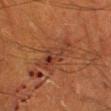<case>
<biopsy_status>not biopsied; imaged during a skin examination</biopsy_status>
<lighting>cross-polarized</lighting>
<lesion_size>
  <long_diameter_mm_approx>4.5</long_diameter_mm_approx>
</lesion_size>
<patient>
  <sex>male</sex>
  <age_approx>60</age_approx>
</patient>
<image>
  <source>total-body photography crop</source>
  <field_of_view_mm>15</field_of_view_mm>
</image>
<site>left lower leg</site>
</case>